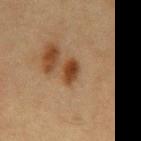Clinical impression:
The lesion was photographed on a routine skin check and not biopsied; there is no pathology result.
Clinical summary:
A 15 mm close-up extracted from a 3D total-body photography capture. The total-body-photography lesion software estimated an outline eccentricity of about 0.8 (0 = round, 1 = elongated) and two-axis asymmetry of about 0.2. And it measured an average lesion color of about L≈35 a*≈18 b*≈30 (CIELAB), roughly 11 lightness units darker than nearby skin, and a normalized lesion–skin contrast near 10. And it measured a within-lesion color-variation index near 3.5/10. The lesion's longest dimension is about 3.5 mm. This is a cross-polarized tile. The lesion is located on the mid back. A male subject, approximately 65 years of age.